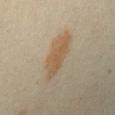The lesion was tiled from a total-body skin photograph and was not biopsied. A close-up tile cropped from a whole-body skin photograph, about 15 mm across. A female subject, aged 33 to 37. Located on the mid back. About 6.5 mm across. Captured under cross-polarized illumination.